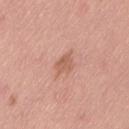Case summary:
- biopsy status — catalogued during a skin exam; not biopsied
- body site — the lower back
- size — about 2.5 mm
- tile lighting — white-light
- imaging modality — 15 mm crop, total-body photography
- automated lesion analysis — a lesion area of about 4 mm², an eccentricity of roughly 0.65, and a shape-asymmetry score of about 0.25 (0 = symmetric); a border-irregularity rating of about 2/10, internal color variation of about 1.5 on a 0–10 scale, and radial color variation of about 0.5; a classifier nevus-likeness of about 5/100 and a detector confidence of about 100 out of 100 that the crop contains a lesion
- patient — male, in their 40s